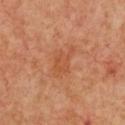Q: Was a biopsy performed?
A: no biopsy performed (imaged during a skin exam)
Q: What is the anatomic site?
A: the front of the torso
Q: What did automated image analysis measure?
A: a footprint of about 7 mm², an eccentricity of roughly 0.8, and two-axis asymmetry of about 0.35; a lesion color around L≈52 a*≈27 b*≈37 in CIELAB and roughly 6 lightness units darker than nearby skin
Q: How was the tile lit?
A: cross-polarized illumination
Q: What kind of image is this?
A: total-body-photography crop, ~15 mm field of view
Q: Lesion size?
A: ≈4 mm
Q: What are the patient's age and sex?
A: female, aged around 40